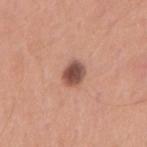| field | value |
|---|---|
| biopsy status | total-body-photography surveillance lesion; no biopsy |
| tile lighting | white-light |
| image source | ~15 mm crop, total-body skin-cancer survey |
| lesion size | ≈2.5 mm |
| patient | male, aged 28 to 32 |
| location | the right upper arm |